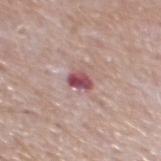Findings:
– notes: no biopsy performed (imaged during a skin exam)
– size: ~2.5 mm (longest diameter)
– acquisition: 15 mm crop, total-body photography
– subject: male, aged approximately 65
– site: the mid back
– image-analysis metrics: a mean CIELAB color near L≈50 a*≈27 b*≈16, a lesion–skin lightness drop of about 15, and a normalized lesion–skin contrast near 10.5; a within-lesion color-variation index near 6.5/10 and peripheral color asymmetry of about 2.5; an automated nevus-likeness rating near 0 out of 100 and a detector confidence of about 100 out of 100 that the crop contains a lesion